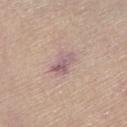- workup · no biopsy performed (imaged during a skin exam)
- subject · female, in their mid-60s
- location · the chest
- acquisition · total-body-photography crop, ~15 mm field of view